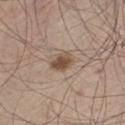| key | value |
|---|---|
| biopsy status | total-body-photography surveillance lesion; no biopsy |
| location | the left thigh |
| subject | male, aged 43–47 |
| illumination | white-light illumination |
| lesion size | ~2.5 mm (longest diameter) |
| image-analysis metrics | an area of roughly 5 mm², an outline eccentricity of about 0.5 (0 = round, 1 = elongated), and a shape-asymmetry score of about 0.25 (0 = symmetric); a border-irregularity rating of about 2/10, a within-lesion color-variation index near 3/10, and a peripheral color-asymmetry measure near 1; an automated nevus-likeness rating near 95 out of 100 |
| acquisition | total-body-photography crop, ~15 mm field of view |